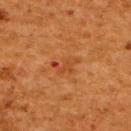The lesion was photographed on a routine skin check and not biopsied; there is no pathology result. A roughly 15 mm field-of-view crop from a total-body skin photograph. The lesion is located on the upper back. A female subject roughly 50 years of age.